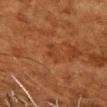This lesion was catalogued during total-body skin photography and was not selected for biopsy.
Cropped from a whole-body photographic skin survey; the tile spans about 15 mm.
Located on the chest.
The recorded lesion diameter is about 2.5 mm.
The tile uses cross-polarized illumination.
A male subject in their 80s.